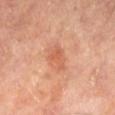Acquisition and patient details:
From the left lower leg. The patient is a male aged 63–67. A 15 mm close-up extracted from a 3D total-body photography capture.